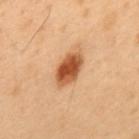biopsy_status: not biopsied; imaged during a skin examination
automated_metrics:
  area_mm2_approx: 8.0
  shape_asymmetry: 0.15
  cielab_L: 43
  cielab_a: 22
  cielab_b: 33
  vs_skin_darker_L: 14.0
  vs_skin_contrast_norm: 11.0
  border_irregularity_0_10: 1.5
  nevus_likeness_0_100: 100
site: mid back
image:
  source: total-body photography crop
  field_of_view_mm: 15
patient:
  sex: male
  age_approx: 55
lesion_size:
  long_diameter_mm_approx: 4.0
lighting: cross-polarized